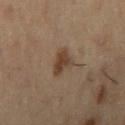Imaged during a routine full-body skin examination; the lesion was not biopsied and no histopathology is available. The lesion's longest dimension is about 3 mm. Imaged with cross-polarized lighting. A male patient, aged 53–57. Located on the right upper arm. A 15 mm crop from a total-body photograph taken for skin-cancer surveillance.